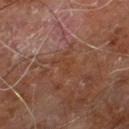illumination: cross-polarized illumination
automated metrics: a mean CIELAB color near L≈38 a*≈21 b*≈29 and a normalized lesion–skin contrast near 4.5; radial color variation of about 0; a classifier nevus-likeness of about 0/100
acquisition: ~15 mm tile from a whole-body skin photo
lesion diameter: about 3 mm
patient: male, approximately 60 years of age
anatomic site: the right leg